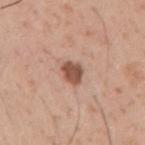<case>
<biopsy_status>not biopsied; imaged during a skin examination</biopsy_status>
<lesion_size>
  <long_diameter_mm_approx>2.5</long_diameter_mm_approx>
</lesion_size>
<site>right upper arm</site>
<patient>
  <sex>male</sex>
  <age_approx>30</age_approx>
</patient>
<image>
  <source>total-body photography crop</source>
  <field_of_view_mm>15</field_of_view_mm>
</image>
</case>